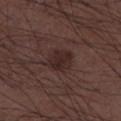Findings:
* image source: 15 mm crop, total-body photography
* location: the right lower leg
* lesion diameter: about 3 mm
* patient: male, aged around 50
* tile lighting: white-light